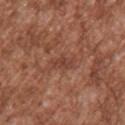Clinical impression: The lesion was photographed on a routine skin check and not biopsied; there is no pathology result. Clinical summary: A male patient, roughly 45 years of age. A roughly 15 mm field-of-view crop from a total-body skin photograph. Imaged with white-light lighting. The lesion is on the upper back.